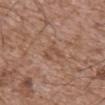This lesion was catalogued during total-body skin photography and was not selected for biopsy.
The tile uses white-light illumination.
A male subject roughly 75 years of age.
An algorithmic analysis of the crop reported a mean CIELAB color near L≈50 a*≈19 b*≈29, about 6 CIELAB-L* units darker than the surrounding skin, and a normalized border contrast of about 5.
A roughly 15 mm field-of-view crop from a total-body skin photograph.
From the abdomen.
Longest diameter approximately 2.5 mm.